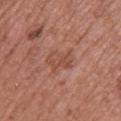Assessment:
The lesion was photographed on a routine skin check and not biopsied; there is no pathology result.
Image and clinical context:
A female subject approximately 60 years of age. About 3.5 mm across. On the upper back. This image is a 15 mm lesion crop taken from a total-body photograph. Imaged with white-light lighting.